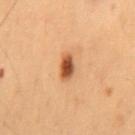Impression: This lesion was catalogued during total-body skin photography and was not selected for biopsy. Clinical summary: This is a cross-polarized tile. A close-up tile cropped from a whole-body skin photograph, about 15 mm across. From the lower back. The lesion's longest dimension is about 3 mm. The patient is a male aged 53 to 57.